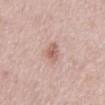This lesion was catalogued during total-body skin photography and was not selected for biopsy.
Imaged with white-light lighting.
The lesion is on the front of the torso.
Longest diameter approximately 2.5 mm.
A male subject aged around 70.
The lesion-visualizer software estimated a lesion color around L≈60 a*≈22 b*≈25 in CIELAB, a lesion–skin lightness drop of about 10, and a normalized border contrast of about 7.
A lesion tile, about 15 mm wide, cut from a 3D total-body photograph.